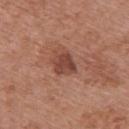follow-up=catalogued during a skin exam; not biopsied
patient=female, in their mid-60s
imaging modality=~15 mm tile from a whole-body skin photo
body site=the upper back